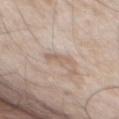Recorded during total-body skin imaging; not selected for excision or biopsy. This is a white-light tile. The lesion is on the chest. A roughly 15 mm field-of-view crop from a total-body skin photograph. The subject is a male aged approximately 65. The recorded lesion diameter is about 3 mm. The total-body-photography lesion software estimated a footprint of about 4 mm² and two-axis asymmetry of about 0.35. The software also gave a border-irregularity index near 4.5/10 and a color-variation rating of about 2/10. The analysis additionally found a classifier nevus-likeness of about 0/100.